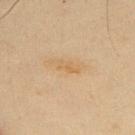workup = catalogued during a skin exam; not biopsied